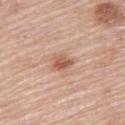No biopsy was performed on this lesion — it was imaged during a full skin examination and was not determined to be concerning. Located on the upper back. A female patient in their mid-60s. A lesion tile, about 15 mm wide, cut from a 3D total-body photograph.